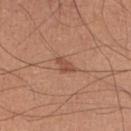  image:
    source: total-body photography crop
    field_of_view_mm: 15
  site: right lower leg
  lesion_size:
    long_diameter_mm_approx: 3.0
  lighting: white-light
  patient:
    sex: male
    age_approx: 55